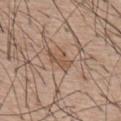Notes:
– imaging modality · 15 mm crop, total-body photography
– location · the upper back
– patient · male, roughly 55 years of age